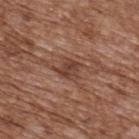Part of a total-body skin-imaging series; this lesion was reviewed on a skin check and was not flagged for biopsy.
A 15 mm close-up tile from a total-body photography series done for melanoma screening.
Located on the upper back.
A male patient roughly 75 years of age.
The lesion-visualizer software estimated roughly 9 lightness units darker than nearby skin and a normalized lesion–skin contrast near 7.5. It also reported a nevus-likeness score of about 10/100 and a lesion-detection confidence of about 95/100.
Longest diameter approximately 3 mm.
Captured under white-light illumination.